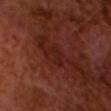Notes:
• notes · no biopsy performed (imaged during a skin exam)
• patient · male, aged 63 to 67
• illumination · cross-polarized illumination
• size · ≈3.5 mm
• image source · ~15 mm crop, total-body skin-cancer survey